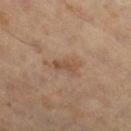Clinical impression:
Part of a total-body skin-imaging series; this lesion was reviewed on a skin check and was not flagged for biopsy.
Background:
Measured at roughly 3 mm in maximum diameter. The total-body-photography lesion software estimated an area of roughly 3.5 mm², an eccentricity of roughly 0.9, and a shape-asymmetry score of about 0.3 (0 = symmetric). It also reported an average lesion color of about L≈45 a*≈17 b*≈28 (CIELAB) and a lesion-to-skin contrast of about 6 (normalized; higher = more distinct). The software also gave a within-lesion color-variation index near 0/10 and a peripheral color-asymmetry measure near 0. And it measured a lesion-detection confidence of about 100/100. The lesion is on the right thigh. A male patient, in their 60s. A lesion tile, about 15 mm wide, cut from a 3D total-body photograph.